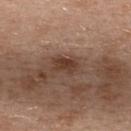Impression:
Recorded during total-body skin imaging; not selected for excision or biopsy.
Clinical summary:
The subject is a female aged 38 to 42. Measured at roughly 3 mm in maximum diameter. The lesion is on the back. The lesion-visualizer software estimated an outline eccentricity of about 0.8 (0 = round, 1 = elongated) and a symmetry-axis asymmetry near 0.3. The analysis additionally found a border-irregularity rating of about 3/10, a within-lesion color-variation index near 2.5/10, and peripheral color asymmetry of about 1. A region of skin cropped from a whole-body photographic capture, roughly 15 mm wide. This is a white-light tile.